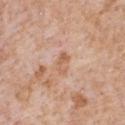This image is a 15 mm lesion crop taken from a total-body photograph.
From the front of the torso.
Captured under white-light illumination.
A male subject, about 65 years old.
Approximately 3 mm at its widest.